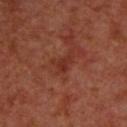The lesion was photographed on a routine skin check and not biopsied; there is no pathology result.
A roughly 15 mm field-of-view crop from a total-body skin photograph.
A male subject, aged approximately 70.
Approximately 2.5 mm at its widest.
Captured under cross-polarized illumination.
On the back.